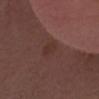Clinical impression: No biopsy was performed on this lesion — it was imaged during a full skin examination and was not determined to be concerning. Image and clinical context: A male patient, in their 40s. A region of skin cropped from a whole-body photographic capture, roughly 15 mm wide. The lesion is on the head or neck. Automated image analysis of the tile measured an automated nevus-likeness rating near 0 out of 100.